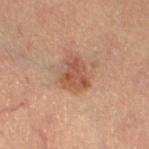The lesion was tiled from a total-body skin photograph and was not biopsied.
Captured under cross-polarized illumination.
The total-body-photography lesion software estimated about 9 CIELAB-L* units darker than the surrounding skin. It also reported border irregularity of about 3.5 on a 0–10 scale, internal color variation of about 3.5 on a 0–10 scale, and radial color variation of about 1. And it measured a nevus-likeness score of about 50/100 and a lesion-detection confidence of about 100/100.
Located on the left thigh.
Cropped from a whole-body photographic skin survey; the tile spans about 15 mm.
The patient is a female aged 58 to 62.